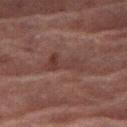This lesion was catalogued during total-body skin photography and was not selected for biopsy. On the right thigh. Cropped from a whole-body photographic skin survey; the tile spans about 15 mm. The subject is a female about 70 years old. Automated image analysis of the tile measured a symmetry-axis asymmetry near 0.5. It also reported a mean CIELAB color near L≈30 a*≈18 b*≈19 and a lesion–skin lightness drop of about 6. Longest diameter approximately 5 mm.